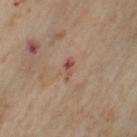The lesion was tiled from a total-body skin photograph and was not biopsied. This is a cross-polarized tile. The lesion is on the left upper arm. Measured at roughly 2.5 mm in maximum diameter. Automated image analysis of the tile measured an area of roughly 2.5 mm² and two-axis asymmetry of about 0.4. The analysis additionally found a border-irregularity index near 3.5/10 and a peripheral color-asymmetry measure near 0. A female patient, aged 53–57. Cropped from a total-body skin-imaging series; the visible field is about 15 mm.